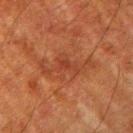* workup — no biopsy performed (imaged during a skin exam)
* lesion diameter — ≈6.5 mm
* image — total-body-photography crop, ~15 mm field of view
* automated metrics — lesion-presence confidence of about 100/100
* location — the arm
* illumination — cross-polarized
* subject — male, aged 78–82